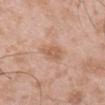Recorded during total-body skin imaging; not selected for excision or biopsy.
Imaged with white-light lighting.
The lesion-visualizer software estimated a footprint of about 6 mm², a shape eccentricity near 0.85, and a shape-asymmetry score of about 0.25 (0 = symmetric). It also reported a lesion color around L≈60 a*≈21 b*≈31 in CIELAB, a lesion–skin lightness drop of about 8, and a normalized border contrast of about 6. The software also gave a color-variation rating of about 2/10 and radial color variation of about 0.5. And it measured a classifier nevus-likeness of about 10/100.
Located on the mid back.
A 15 mm close-up extracted from a 3D total-body photography capture.
A male subject, aged 48–52.
The recorded lesion diameter is about 4 mm.